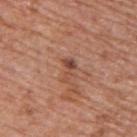Clinical impression: Part of a total-body skin-imaging series; this lesion was reviewed on a skin check and was not flagged for biopsy.